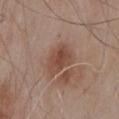  biopsy_status: not biopsied; imaged during a skin examination
  automated_metrics:
    cielab_L: 47
    cielab_a: 20
    cielab_b: 27
    vs_skin_contrast_norm: 7.5
    nevus_likeness_0_100: 70
  image:
    source: total-body photography crop
    field_of_view_mm: 15
  patient:
    sex: male
    age_approx: 55
  lighting: white-light
  site: mid back